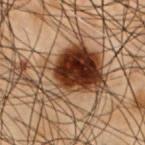  biopsy_status: not biopsied; imaged during a skin examination
  lesion_size:
    long_diameter_mm_approx: 8.5
  site: front of the torso
  lighting: cross-polarized
  image:
    source: total-body photography crop
    field_of_view_mm: 15
  patient:
    sex: male
    age_approx: 55